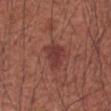Assessment: This lesion was catalogued during total-body skin photography and was not selected for biopsy. Acquisition and patient details: Cropped from a whole-body photographic skin survey; the tile spans about 15 mm. The tile uses white-light illumination. On the right forearm. The lesion's longest dimension is about 3.5 mm. The lesion-visualizer software estimated border irregularity of about 3.5 on a 0–10 scale, internal color variation of about 2 on a 0–10 scale, and a peripheral color-asymmetry measure near 0.5. And it measured lesion-presence confidence of about 100/100. A male subject aged approximately 60.